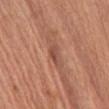Q: Is there a histopathology result?
A: imaged on a skin check; not biopsied
Q: Who is the patient?
A: male, aged approximately 65
Q: Lesion location?
A: the front of the torso
Q: How was this image acquired?
A: 15 mm crop, total-body photography
Q: How large is the lesion?
A: about 3 mm
Q: How was the tile lit?
A: white-light illumination